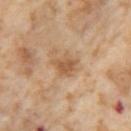Part of a total-body skin-imaging series; this lesion was reviewed on a skin check and was not flagged for biopsy. The recorded lesion diameter is about 3 mm. Cropped from a total-body skin-imaging series; the visible field is about 15 mm. The patient is a female about 55 years old. On the left thigh. Captured under cross-polarized illumination.